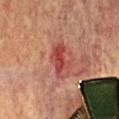Captured during whole-body skin photography for melanoma surveillance; the lesion was not biopsied. A female patient, about 80 years old. The lesion's longest dimension is about 4.5 mm. The total-body-photography lesion software estimated an average lesion color of about L≈42 a*≈31 b*≈26 (CIELAB), a lesion–skin lightness drop of about 10, and a normalized border contrast of about 8. The analysis additionally found a border-irregularity rating of about 4.5/10, a within-lesion color-variation index near 3.5/10, and peripheral color asymmetry of about 1. And it measured a lesion-detection confidence of about 100/100. Located on the front of the torso. A close-up tile cropped from a whole-body skin photograph, about 15 mm across.